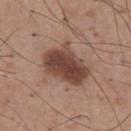Notes:
- workup: imaged on a skin check; not biopsied
- anatomic site: the abdomen
- lesion size: ≈5.5 mm
- image source: total-body-photography crop, ~15 mm field of view
- automated lesion analysis: an area of roughly 18 mm² and a symmetry-axis asymmetry near 0.2; a border-irregularity index near 2.5/10, a color-variation rating of about 4/10, and a peripheral color-asymmetry measure near 1.5
- patient: male, in their mid-60s